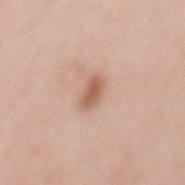follow-up=catalogued during a skin exam; not biopsied | imaging modality=total-body-photography crop, ~15 mm field of view | lesion size=≈3.5 mm | tile lighting=white-light illumination | location=the mid back | patient=female, aged 38 to 42.